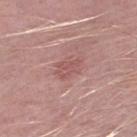Context:
Approximately 3 mm at its widest. The patient is a male aged approximately 50. This is a white-light tile. On the right thigh. This image is a 15 mm lesion crop taken from a total-body photograph.